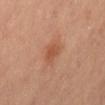follow-up: no biopsy performed (imaged during a skin exam) | image: total-body-photography crop, ~15 mm field of view | diameter: ~2.5 mm (longest diameter) | TBP lesion metrics: a lesion area of about 4.5 mm² and two-axis asymmetry of about 0.3; a classifier nevus-likeness of about 55/100 and lesion-presence confidence of about 100/100 | location: the mid back | subject: female | illumination: cross-polarized.